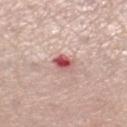Q: Was this lesion biopsied?
A: catalogued during a skin exam; not biopsied
Q: How was this image acquired?
A: ~15 mm crop, total-body skin-cancer survey
Q: What are the patient's age and sex?
A: female, roughly 65 years of age
Q: What is the anatomic site?
A: the right lower leg